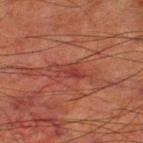Impression: Captured during whole-body skin photography for melanoma surveillance; the lesion was not biopsied. Context: From the left thigh. Imaged with cross-polarized lighting. Measured at roughly 3 mm in maximum diameter. The subject is a male in their 80s. A close-up tile cropped from a whole-body skin photograph, about 15 mm across.